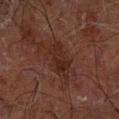The lesion was photographed on a routine skin check and not biopsied; there is no pathology result.
A lesion tile, about 15 mm wide, cut from a 3D total-body photograph.
Automated image analysis of the tile measured an area of roughly 7.5 mm², an eccentricity of roughly 0.9, and a symmetry-axis asymmetry near 0.3. The analysis additionally found a border-irregularity rating of about 4.5/10, a color-variation rating of about 2.5/10, and peripheral color asymmetry of about 1.
This is a cross-polarized tile.
Approximately 4.5 mm at its widest.
On the arm.
A male subject aged 68 to 72.